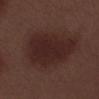The lesion's longest dimension is about 8 mm. Located on the left lower leg. The patient is a male aged 68–72. An algorithmic analysis of the crop reported an area of roughly 27 mm², a shape eccentricity near 0.85, and a shape-asymmetry score of about 0.25 (0 = symmetric). The analysis additionally found border irregularity of about 3 on a 0–10 scale and a within-lesion color-variation index near 2.5/10. Captured under white-light illumination. A close-up tile cropped from a whole-body skin photograph, about 15 mm across.